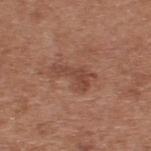No biopsy was performed on this lesion — it was imaged during a full skin examination and was not determined to be concerning. Cropped from a whole-body photographic skin survey; the tile spans about 15 mm. On the upper back. A male patient, in their mid- to late 50s.